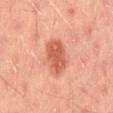This lesion was catalogued during total-body skin photography and was not selected for biopsy. Measured at roughly 5 mm in maximum diameter. On the mid back. Automated image analysis of the tile measured a lesion area of about 9.5 mm², an outline eccentricity of about 0.9 (0 = round, 1 = elongated), and two-axis asymmetry of about 0.2. And it measured about 10 CIELAB-L* units darker than the surrounding skin and a normalized lesion–skin contrast near 8. And it measured a color-variation rating of about 2/10 and peripheral color asymmetry of about 1. A lesion tile, about 15 mm wide, cut from a 3D total-body photograph. The subject is a male approximately 45 years of age. This is a cross-polarized tile.